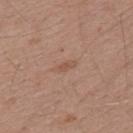<case>
<patient>
  <sex>male</sex>
  <age_approx>55</age_approx>
</patient>
<site>mid back</site>
<lesion_size>
  <long_diameter_mm_approx>2.5</long_diameter_mm_approx>
</lesion_size>
<lighting>white-light</lighting>
<image>
  <source>total-body photography crop</source>
  <field_of_view_mm>15</field_of_view_mm>
</image>
</case>